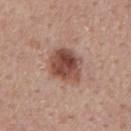A male patient aged around 60. Imaged with white-light lighting. A roughly 15 mm field-of-view crop from a total-body skin photograph. Located on the back.